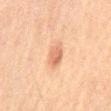Case summary:
• follow-up: imaged on a skin check; not biopsied
• lesion size: ~3 mm (longest diameter)
• imaging modality: total-body-photography crop, ~15 mm field of view
• tile lighting: cross-polarized illumination
• automated metrics: a border-irregularity rating of about 1.5/10, internal color variation of about 3 on a 0–10 scale, and peripheral color asymmetry of about 1
• location: the abdomen
• subject: male, approximately 60 years of age